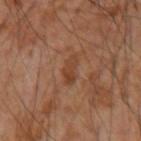• patient · male, in their mid- to late 50s
• size · ≈3 mm
• anatomic site · the arm
• image-analysis metrics · an area of roughly 4 mm² and an eccentricity of roughly 0.9; an average lesion color of about L≈42 a*≈22 b*≈32 (CIELAB), about 7 CIELAB-L* units darker than the surrounding skin, and a normalized border contrast of about 6.5; a border-irregularity rating of about 4/10 and internal color variation of about 3 on a 0–10 scale
• image · ~15 mm tile from a whole-body skin photo
• tile lighting · cross-polarized illumination